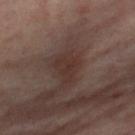The lesion was tiled from a total-body skin photograph and was not biopsied. Approximately 3.5 mm at its widest. Automated tile analysis of the lesion measured a shape eccentricity near 0.7. The software also gave a lesion color around L≈33 a*≈17 b*≈21 in CIELAB, about 6 CIELAB-L* units darker than the surrounding skin, and a normalized border contrast of about 6.5. It also reported an automated nevus-likeness rating near 5 out of 100 and lesion-presence confidence of about 100/100. Captured under cross-polarized illumination. On the right thigh. A female subject aged 53–57. A 15 mm close-up extracted from a 3D total-body photography capture.